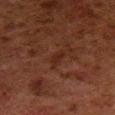{
  "biopsy_status": "not biopsied; imaged during a skin examination",
  "site": "left lower leg",
  "patient": {
    "sex": "male",
    "age_approx": 80
  },
  "image": {
    "source": "total-body photography crop",
    "field_of_view_mm": 15
  },
  "automated_metrics": {
    "border_irregularity_0_10": 4.0,
    "color_variation_0_10": 0.0,
    "peripheral_color_asymmetry": 0.0
  }
}